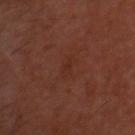notes — catalogued during a skin exam; not biopsied | patient — male, approximately 65 years of age | lighting — cross-polarized | diameter — about 2 mm | body site — the head or neck | acquisition — ~15 mm tile from a whole-body skin photo.